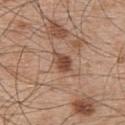Q: Was a biopsy performed?
A: total-body-photography surveillance lesion; no biopsy
Q: How was this image acquired?
A: ~15 mm crop, total-body skin-cancer survey
Q: How was the tile lit?
A: white-light illumination
Q: What is the anatomic site?
A: the upper back
Q: Patient demographics?
A: male, aged 48–52
Q: What did automated image analysis measure?
A: a border-irregularity rating of about 2.5/10, a within-lesion color-variation index near 4/10, and a peripheral color-asymmetry measure near 1.5